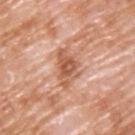Impression: No biopsy was performed on this lesion — it was imaged during a full skin examination and was not determined to be concerning. Background: Imaged with white-light lighting. The patient is a male aged 58–62. A 15 mm crop from a total-body photograph taken for skin-cancer surveillance. From the back.